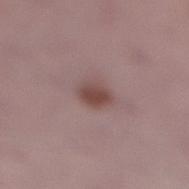patient:
  sex: female
  age_approx: 40
lighting: white-light
lesion_size:
  long_diameter_mm_approx: 3.0
site: right lower leg
image:
  source: total-body photography crop
  field_of_view_mm: 15
automated_metrics:
  border_irregularity_0_10: 1.5
  color_variation_0_10: 2.5
  peripheral_color_asymmetry: 1.0
  nevus_likeness_0_100: 95
  lesion_detection_confidence_0_100: 100